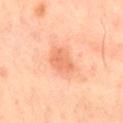No biopsy was performed on this lesion — it was imaged during a full skin examination and was not determined to be concerning.
A male subject, roughly 65 years of age.
Captured under cross-polarized illumination.
A 15 mm close-up extracted from a 3D total-body photography capture.
The recorded lesion diameter is about 3.5 mm.
Automated image analysis of the tile measured a lesion area of about 7.5 mm² and a symmetry-axis asymmetry near 0.2. The software also gave a normalized lesion–skin contrast near 5.5.
From the lower back.